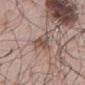Q: Was a biopsy performed?
A: catalogued during a skin exam; not biopsied
Q: What is the anatomic site?
A: the mid back
Q: What are the patient's age and sex?
A: male, in their mid- to late 60s
Q: How was this image acquired?
A: ~15 mm tile from a whole-body skin photo